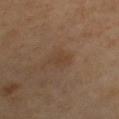workup: imaged on a skin check; not biopsied
TBP lesion metrics: an outline eccentricity of about 0.85 (0 = round, 1 = elongated); a border-irregularity index near 4/10, a color-variation rating of about 1/10, and a peripheral color-asymmetry measure near 0.5
body site: the chest
image source: 15 mm crop, total-body photography
patient: female, aged 38–42
lighting: cross-polarized
diameter: ~3 mm (longest diameter)